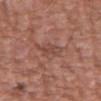The lesion was photographed on a routine skin check and not biopsied; there is no pathology result. Automated tile analysis of the lesion measured an automated nevus-likeness rating near 0 out of 100 and lesion-presence confidence of about 100/100. A 15 mm close-up tile from a total-body photography series done for melanoma screening. Located on the left upper arm. Captured under white-light illumination. A male subject, in their mid-70s.